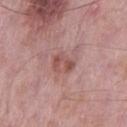Part of a total-body skin-imaging series; this lesion was reviewed on a skin check and was not flagged for biopsy. Measured at roughly 3 mm in maximum diameter. From the left thigh. A close-up tile cropped from a whole-body skin photograph, about 15 mm across. The patient is a male aged approximately 55. This is a white-light tile.